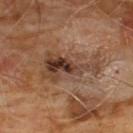| field | value |
|---|---|
| tile lighting | cross-polarized illumination |
| patient | male, about 60 years old |
| automated lesion analysis | a lesion color around L≈39 a*≈17 b*≈27 in CIELAB, a lesion–skin lightness drop of about 10, and a normalized lesion–skin contrast near 8.5; border irregularity of about 7.5 on a 0–10 scale, a color-variation rating of about 9/10, and peripheral color asymmetry of about 3 |
| image | total-body-photography crop, ~15 mm field of view |
| size | ≈7 mm |
| location | the chest |